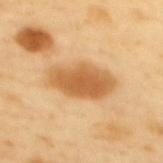Q: Is there a histopathology result?
A: imaged on a skin check; not biopsied
Q: What kind of image is this?
A: total-body-photography crop, ~15 mm field of view
Q: How large is the lesion?
A: ~6.5 mm (longest diameter)
Q: What lighting was used for the tile?
A: cross-polarized illumination
Q: Where on the body is the lesion?
A: the mid back
Q: What are the patient's age and sex?
A: female, aged 53–57
Q: Automated lesion metrics?
A: a mean CIELAB color near L≈62 a*≈22 b*≈44 and a normalized lesion–skin contrast near 8.5; a border-irregularity rating of about 2.5/10, internal color variation of about 3.5 on a 0–10 scale, and a peripheral color-asymmetry measure near 1; a nevus-likeness score of about 70/100 and lesion-presence confidence of about 100/100